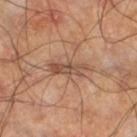follow-up = no biopsy performed (imaged during a skin exam)
subject = male, approximately 60 years of age
image source = ~15 mm crop, total-body skin-cancer survey
site = the right thigh
lesion size = ≈5 mm
illumination = cross-polarized illumination